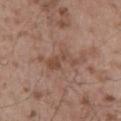The lesion was photographed on a routine skin check and not biopsied; there is no pathology result. A roughly 15 mm field-of-view crop from a total-body skin photograph. The lesion's longest dimension is about 4 mm. From the lower back. A male subject, approximately 55 years of age.